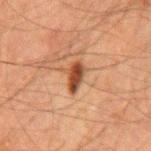{"biopsy_status": "not biopsied; imaged during a skin examination", "image": {"source": "total-body photography crop", "field_of_view_mm": 15}, "patient": {"sex": "male", "age_approx": 60}, "site": "mid back"}